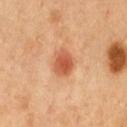workup: no biopsy performed (imaged during a skin exam); imaging modality: ~15 mm crop, total-body skin-cancer survey; tile lighting: cross-polarized; site: the chest; lesion size: ≈3.5 mm; patient: male, aged 48 to 52.